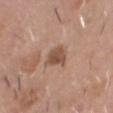Image and clinical context:
A male subject aged around 50. Located on the head or neck. A roughly 15 mm field-of-view crop from a total-body skin photograph. The lesion's longest dimension is about 3.5 mm. The tile uses white-light illumination.